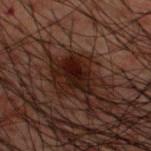This image is a 15 mm lesion crop taken from a total-body photograph.
Automated tile analysis of the lesion measured an average lesion color of about L≈12 a*≈15 b*≈14 (CIELAB), a lesion–skin lightness drop of about 8, and a normalized lesion–skin contrast near 12.
A male patient aged 48–52.
From the upper back.
This is a cross-polarized tile.
The lesion's longest dimension is about 4 mm.